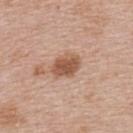This lesion was catalogued during total-body skin photography and was not selected for biopsy.
The tile uses white-light illumination.
The lesion's longest dimension is about 3.5 mm.
Automated tile analysis of the lesion measured a lesion area of about 7 mm². The software also gave a mean CIELAB color near L≈54 a*≈21 b*≈31, a lesion–skin lightness drop of about 13, and a lesion-to-skin contrast of about 9 (normalized; higher = more distinct). The analysis additionally found a border-irregularity index near 1.5/10, a color-variation rating of about 2.5/10, and peripheral color asymmetry of about 1. It also reported an automated nevus-likeness rating near 80 out of 100.
The patient is a female roughly 50 years of age.
A close-up tile cropped from a whole-body skin photograph, about 15 mm across.
The lesion is located on the upper back.